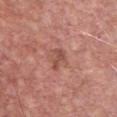workup: catalogued during a skin exam; not biopsied
subject: male, in their mid- to late 50s
diameter: ~3 mm (longest diameter)
anatomic site: the chest
image: total-body-photography crop, ~15 mm field of view